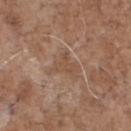tile lighting = white-light illumination | acquisition = total-body-photography crop, ~15 mm field of view | lesion size = ~3.5 mm (longest diameter) | subject = male, aged 68–72 | location = the chest.